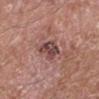Recorded during total-body skin imaging; not selected for excision or biopsy. Automated tile analysis of the lesion measured a footprint of about 6.5 mm² and an eccentricity of roughly 0.75. It also reported a lesion color around L≈44 a*≈21 b*≈21 in CIELAB, about 12 CIELAB-L* units darker than the surrounding skin, and a lesion-to-skin contrast of about 9 (normalized; higher = more distinct). The analysis additionally found a border-irregularity index near 3/10, a color-variation rating of about 7/10, and peripheral color asymmetry of about 2.5. Located on the right lower leg. Captured under white-light illumination. A roughly 15 mm field-of-view crop from a total-body skin photograph. A male patient, about 60 years old.